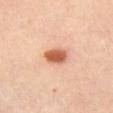Case summary:
– tile lighting · cross-polarized illumination
– subject · female, about 40 years old
– automated metrics · a border-irregularity rating of about 1.5/10, a within-lesion color-variation index near 4/10, and peripheral color asymmetry of about 1.5
– location · the front of the torso
– acquisition · total-body-photography crop, ~15 mm field of view
– size · ~3.5 mm (longest diameter)